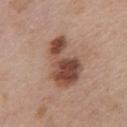Assessment: No biopsy was performed on this lesion — it was imaged during a full skin examination and was not determined to be concerning. Acquisition and patient details: A female patient, approximately 45 years of age. About 6.5 mm across. This image is a 15 mm lesion crop taken from a total-body photograph. On the chest. The lesion-visualizer software estimated a mean CIELAB color near L≈48 a*≈20 b*≈27, a lesion–skin lightness drop of about 14, and a lesion-to-skin contrast of about 10 (normalized; higher = more distinct). And it measured border irregularity of about 3.5 on a 0–10 scale, a within-lesion color-variation index near 8.5/10, and radial color variation of about 3.